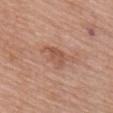follow-up: total-body-photography surveillance lesion; no biopsy | patient: female, aged around 65 | lesion size: ~3.5 mm (longest diameter) | location: the upper back | lighting: white-light | automated lesion analysis: an eccentricity of roughly 0.85 and a shape-asymmetry score of about 0.4 (0 = symmetric); an automated nevus-likeness rating near 5 out of 100 and a lesion-detection confidence of about 100/100 | image source: ~15 mm crop, total-body skin-cancer survey.